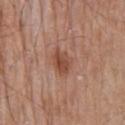Assessment:
Captured during whole-body skin photography for melanoma surveillance; the lesion was not biopsied.
Background:
Longest diameter approximately 3 mm. Cropped from a total-body skin-imaging series; the visible field is about 15 mm. Automated image analysis of the tile measured roughly 10 lightness units darker than nearby skin. And it measured a border-irregularity rating of about 2.5/10, a color-variation rating of about 3.5/10, and a peripheral color-asymmetry measure near 1. On the back. A male patient about 80 years old. Captured under white-light illumination.